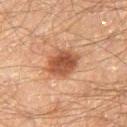| feature | finding |
|---|---|
| notes | no biopsy performed (imaged during a skin exam) |
| lesion diameter | about 4 mm |
| image-analysis metrics | an area of roughly 9 mm², a shape eccentricity near 0.65, and a symmetry-axis asymmetry near 0.2; a normalized lesion–skin contrast near 9.5; a classifier nevus-likeness of about 90/100 and a detector confidence of about 100 out of 100 that the crop contains a lesion |
| site | the right thigh |
| patient | male, approximately 60 years of age |
| imaging modality | total-body-photography crop, ~15 mm field of view |